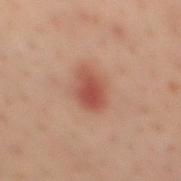This lesion was catalogued during total-body skin photography and was not selected for biopsy. A 15 mm close-up extracted from a 3D total-body photography capture. The lesion-visualizer software estimated an average lesion color of about L≈40 a*≈22 b*≈24 (CIELAB), a lesion–skin lightness drop of about 9, and a lesion-to-skin contrast of about 7.5 (normalized; higher = more distinct). The software also gave a border-irregularity index near 2/10, a color-variation rating of about 4/10, and radial color variation of about 1.5. And it measured a detector confidence of about 100 out of 100 that the crop contains a lesion. The tile uses cross-polarized illumination. Located on the mid back. The lesion's longest dimension is about 4.5 mm. A male patient, approximately 40 years of age.